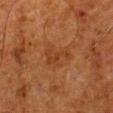The lesion was photographed on a routine skin check and not biopsied; there is no pathology result.
Captured under cross-polarized illumination.
About 4 mm across.
On the left lower leg.
A roughly 15 mm field-of-view crop from a total-body skin photograph.
A male subject aged around 80.